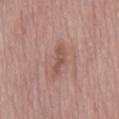The lesion was photographed on a routine skin check and not biopsied; there is no pathology result.
An algorithmic analysis of the crop reported an area of roughly 5 mm², an eccentricity of roughly 0.9, and a symmetry-axis asymmetry near 0.4. The software also gave an average lesion color of about L≈53 a*≈21 b*≈24 (CIELAB), a lesion–skin lightness drop of about 8, and a normalized border contrast of about 6.
Located on the mid back.
A male subject, in their mid- to late 80s or older.
A 15 mm close-up extracted from a 3D total-body photography capture.